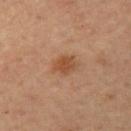<record>
<biopsy_status>not biopsied; imaged during a skin examination</biopsy_status>
<automated_metrics>
  <area_mm2_approx>4.5</area_mm2_approx>
</automated_metrics>
<image>
  <source>total-body photography crop</source>
  <field_of_view_mm>15</field_of_view_mm>
</image>
<site>left upper arm</site>
<patient>
  <sex>female</sex>
  <age_approx>45</age_approx>
</patient>
</record>